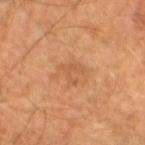Measured at roughly 3 mm in maximum diameter. A male subject, aged 63 to 67. A lesion tile, about 15 mm wide, cut from a 3D total-body photograph. This is a cross-polarized tile. The total-body-photography lesion software estimated a mean CIELAB color near L≈55 a*≈23 b*≈38 and roughly 6 lightness units darker than nearby skin. And it measured a nevus-likeness score of about 0/100 and a lesion-detection confidence of about 100/100.